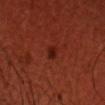Findings:
- follow-up · imaged on a skin check; not biopsied
- lesion diameter · about 2 mm
- anatomic site · the head or neck
- acquisition · total-body-photography crop, ~15 mm field of view
- patient · male, about 35 years old